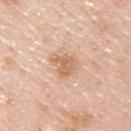Clinical impression:
Recorded during total-body skin imaging; not selected for excision or biopsy.
Acquisition and patient details:
A 15 mm close-up tile from a total-body photography series done for melanoma screening. About 3.5 mm across. The patient is a male aged around 45. Imaged with white-light lighting. Automated tile analysis of the lesion measured border irregularity of about 2.5 on a 0–10 scale, a color-variation rating of about 3/10, and a peripheral color-asymmetry measure near 1. The software also gave a nevus-likeness score of about 5/100. On the upper back.